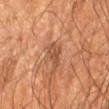The lesion was photographed on a routine skin check and not biopsied; there is no pathology result. The total-body-photography lesion software estimated a border-irregularity rating of about 5/10. The lesion is located on the left forearm. The tile uses cross-polarized illumination. The recorded lesion diameter is about 3.5 mm. A 15 mm close-up tile from a total-body photography series done for melanoma screening. A male patient, aged around 55.